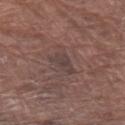Findings:
– workup · catalogued during a skin exam; not biopsied
– location · the left upper arm
– size · about 3.5 mm
– acquisition · ~15 mm tile from a whole-body skin photo
– patient · male, aged around 80